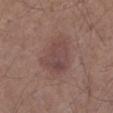Assessment:
Recorded during total-body skin imaging; not selected for excision or biopsy.
Context:
The lesion's longest dimension is about 5 mm. The patient is a male about 75 years old. A close-up tile cropped from a whole-body skin photograph, about 15 mm across. Captured under white-light illumination. From the left lower leg. An algorithmic analysis of the crop reported a footprint of about 16 mm², an eccentricity of roughly 0.65, and a symmetry-axis asymmetry near 0.25. The software also gave an average lesion color of about L≈45 a*≈19 b*≈20 (CIELAB) and a lesion-to-skin contrast of about 6 (normalized; higher = more distinct).